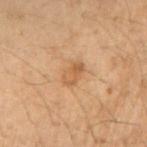Clinical impression: This lesion was catalogued during total-body skin photography and was not selected for biopsy. Acquisition and patient details: The subject is a female aged around 55. Cropped from a whole-body photographic skin survey; the tile spans about 15 mm. Measured at roughly 3 mm in maximum diameter. Automated image analysis of the tile measured a footprint of about 4.5 mm², an outline eccentricity of about 0.75 (0 = round, 1 = elongated), and a shape-asymmetry score of about 0.2 (0 = symmetric). It also reported a mean CIELAB color near L≈48 a*≈18 b*≈33, roughly 7 lightness units darker than nearby skin, and a normalized border contrast of about 6. The software also gave a border-irregularity index near 2/10, a color-variation rating of about 3.5/10, and a peripheral color-asymmetry measure near 1.5. It also reported a classifier nevus-likeness of about 15/100 and a detector confidence of about 100 out of 100 that the crop contains a lesion. Captured under cross-polarized illumination. The lesion is on the arm.